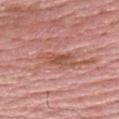Impression: No biopsy was performed on this lesion — it was imaged during a full skin examination and was not determined to be concerning. Image and clinical context: A roughly 15 mm field-of-view crop from a total-body skin photograph. The lesion is located on the upper back. The recorded lesion diameter is about 5 mm. A male patient approximately 65 years of age.